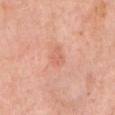| key | value |
|---|---|
| follow-up | imaged on a skin check; not biopsied |
| anatomic site | the right upper arm |
| patient | female, aged around 50 |
| acquisition | 15 mm crop, total-body photography |
| automated metrics | a footprint of about 4 mm², an eccentricity of roughly 0.65, and two-axis asymmetry of about 0.35; a color-variation rating of about 2/10 and a peripheral color-asymmetry measure near 0.5 |
| lesion diameter | about 2.5 mm |
| lighting | white-light illumination |